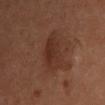Q: What kind of image is this?
A: ~15 mm crop, total-body skin-cancer survey
Q: How large is the lesion?
A: ~5 mm (longest diameter)
Q: Lesion location?
A: the chest
Q: Illumination type?
A: cross-polarized illumination
Q: What did automated image analysis measure?
A: an automated nevus-likeness rating near 20 out of 100
Q: Patient demographics?
A: female, in their mid- to late 40s